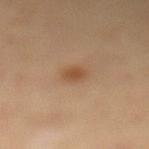biopsy_status: not biopsied; imaged during a skin examination
image:
  source: total-body photography crop
  field_of_view_mm: 15
automated_metrics:
  nevus_likeness_0_100: 80
  lesion_detection_confidence_0_100: 100
patient:
  sex: female
  age_approx: 40
site: left lower leg
lesion_size:
  long_diameter_mm_approx: 2.0